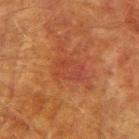biopsy status: imaged on a skin check; not biopsied | subject: male, aged approximately 75 | lighting: cross-polarized | anatomic site: the right upper arm | size: ~3.5 mm (longest diameter) | automated lesion analysis: an area of roughly 7 mm² and a shape-asymmetry score of about 0.2 (0 = symmetric); a border-irregularity index near 2/10 and peripheral color asymmetry of about 1 | imaging modality: ~15 mm crop, total-body skin-cancer survey.